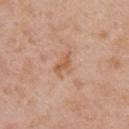Assessment: No biopsy was performed on this lesion — it was imaged during a full skin examination and was not determined to be concerning. Context: The lesion is on the right upper arm. The lesion-visualizer software estimated a lesion area of about 4 mm² and an eccentricity of roughly 0.85. A female patient, aged 38 to 42. Longest diameter approximately 3.5 mm. A region of skin cropped from a whole-body photographic capture, roughly 15 mm wide.